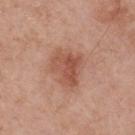Q: Is there a histopathology result?
A: imaged on a skin check; not biopsied
Q: What is the imaging modality?
A: total-body-photography crop, ~15 mm field of view
Q: What is the anatomic site?
A: the upper back
Q: How was the tile lit?
A: white-light illumination
Q: What are the patient's age and sex?
A: male, roughly 55 years of age